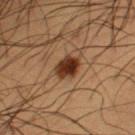notes: total-body-photography surveillance lesion; no biopsy
automated lesion analysis: a border-irregularity index near 2/10, a color-variation rating of about 4/10, and radial color variation of about 1.5; a classifier nevus-likeness of about 100/100 and lesion-presence confidence of about 100/100
size: about 3.5 mm
tile lighting: cross-polarized illumination
imaging modality: ~15 mm crop, total-body skin-cancer survey
anatomic site: the arm
subject: male, approximately 50 years of age